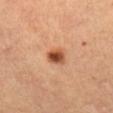Part of a total-body skin-imaging series; this lesion was reviewed on a skin check and was not flagged for biopsy.
From the left thigh.
The patient is female.
Captured under cross-polarized illumination.
A 15 mm crop from a total-body photograph taken for skin-cancer surveillance.